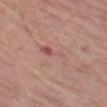Q: Was a biopsy performed?
A: total-body-photography surveillance lesion; no biopsy
Q: What kind of image is this?
A: 15 mm crop, total-body photography
Q: What are the patient's age and sex?
A: male, about 75 years old
Q: Lesion location?
A: the left thigh
Q: Automated lesion metrics?
A: a lesion color around L≈55 a*≈23 b*≈23 in CIELAB, a lesion–skin lightness drop of about 6, and a normalized lesion–skin contrast near 4.5
Q: How was the tile lit?
A: white-light illumination